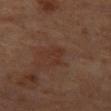Recorded during total-body skin imaging; not selected for excision or biopsy.
Imaged with cross-polarized lighting.
Cropped from a whole-body photographic skin survey; the tile spans about 15 mm.
Longest diameter approximately 2.5 mm.
A male subject approximately 60 years of age.
The lesion-visualizer software estimated a lesion color around L≈29 a*≈18 b*≈23 in CIELAB, a lesion–skin lightness drop of about 4, and a normalized lesion–skin contrast near 4.5. It also reported border irregularity of about 5 on a 0–10 scale and a peripheral color-asymmetry measure near 0. It also reported an automated nevus-likeness rating near 0 out of 100.
The lesion is on the left thigh.